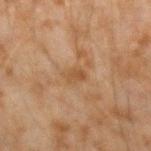Captured during whole-body skin photography for melanoma surveillance; the lesion was not biopsied. A male patient aged around 45. This image is a 15 mm lesion crop taken from a total-body photograph. The lesion-visualizer software estimated a footprint of about 3.5 mm² and a shape eccentricity near 0.75. The software also gave a lesion color around L≈41 a*≈16 b*≈30 in CIELAB, a lesion–skin lightness drop of about 6, and a normalized border contrast of about 6. The analysis additionally found a border-irregularity rating of about 2.5/10, a within-lesion color-variation index near 2/10, and a peripheral color-asymmetry measure near 0.5. This is a cross-polarized tile. The lesion is located on the left forearm.